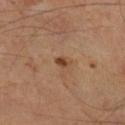Clinical impression:
This lesion was catalogued during total-body skin photography and was not selected for biopsy.
Background:
The subject is a male approximately 65 years of age. On the right lower leg. Cropped from a total-body skin-imaging series; the visible field is about 15 mm.